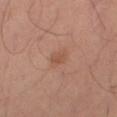notes = imaged on a skin check; not biopsied | image = ~15 mm tile from a whole-body skin photo | anatomic site = the right thigh | size = ~3 mm (longest diameter) | patient = male, aged 63 to 67 | illumination = cross-polarized | TBP lesion metrics = roughly 6 lightness units darker than nearby skin and a normalized border contrast of about 5; a border-irregularity index near 2/10, a within-lesion color-variation index near 1.5/10, and radial color variation of about 0.5.